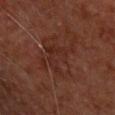Q: Was this lesion biopsied?
A: imaged on a skin check; not biopsied
Q: What is the lesion's diameter?
A: ~6.5 mm (longest diameter)
Q: Where on the body is the lesion?
A: the chest
Q: What are the patient's age and sex?
A: male, aged 63–67
Q: Illumination type?
A: cross-polarized illumination
Q: What is the imaging modality?
A: 15 mm crop, total-body photography